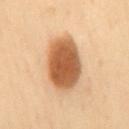Context: The lesion is located on the mid back. Approximately 6.5 mm at its widest. A roughly 15 mm field-of-view crop from a total-body skin photograph. The lesion-visualizer software estimated a footprint of about 23 mm² and a shape eccentricity near 0.7. The analysis additionally found an average lesion color of about L≈51 a*≈20 b*≈34 (CIELAB), a lesion–skin lightness drop of about 16, and a normalized border contrast of about 11. The software also gave a classifier nevus-likeness of about 100/100 and a lesion-detection confidence of about 100/100. A female subject, aged approximately 60.